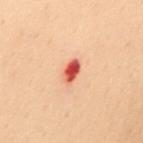This lesion was catalogued during total-body skin photography and was not selected for biopsy. From the mid back. A roughly 15 mm field-of-view crop from a total-body skin photograph. The tile uses cross-polarized illumination. Approximately 2.5 mm at its widest. The patient is a female aged approximately 60.